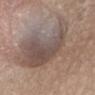This lesion was catalogued during total-body skin photography and was not selected for biopsy. A female subject, in their 20s. Measured at roughly 13.5 mm in maximum diameter. A close-up tile cropped from a whole-body skin photograph, about 15 mm across. On the lower back.